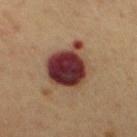Captured during whole-body skin photography for melanoma surveillance; the lesion was not biopsied. A male patient, aged around 60. Cropped from a total-body skin-imaging series; the visible field is about 15 mm. Located on the front of the torso.